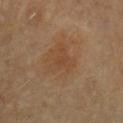Q: Was a biopsy performed?
A: imaged on a skin check; not biopsied
Q: What are the patient's age and sex?
A: female, about 50 years old
Q: How was the tile lit?
A: cross-polarized illumination
Q: Lesion size?
A: ≈1.5 mm
Q: What kind of image is this?
A: ~15 mm tile from a whole-body skin photo
Q: Where on the body is the lesion?
A: the chest